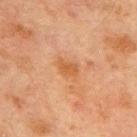– follow-up: total-body-photography surveillance lesion; no biopsy
– lesion size: ~3 mm (longest diameter)
– image: total-body-photography crop, ~15 mm field of view
– patient: male, in their mid- to late 60s
– automated metrics: a lesion area of about 4.5 mm², an eccentricity of roughly 0.8, and a shape-asymmetry score of about 0.35 (0 = symmetric); a mean CIELAB color near L≈46 a*≈21 b*≈34, about 6 CIELAB-L* units darker than the surrounding skin, and a normalized border contrast of about 6; a border-irregularity index near 3.5/10, internal color variation of about 1 on a 0–10 scale, and peripheral color asymmetry of about 0.5; an automated nevus-likeness rating near 0 out of 100
– location: the chest